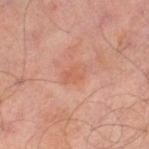* biopsy status · catalogued during a skin exam; not biopsied
* size · ~3 mm (longest diameter)
* illumination · cross-polarized
* patient · male, aged 63 to 67
* location · the left thigh
* image · 15 mm crop, total-body photography